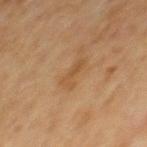Imaged during a routine full-body skin examination; the lesion was not biopsied and no histopathology is available. Located on the mid back. A roughly 15 mm field-of-view crop from a total-body skin photograph. A male patient, roughly 65 years of age. An algorithmic analysis of the crop reported a footprint of about 4.5 mm² and two-axis asymmetry of about 0.4. The software also gave a mean CIELAB color near L≈44 a*≈17 b*≈33, about 6 CIELAB-L* units darker than the surrounding skin, and a lesion-to-skin contrast of about 5.5 (normalized; higher = more distinct). The analysis additionally found a border-irregularity rating of about 5/10, a color-variation rating of about 1.5/10, and radial color variation of about 0.5. The analysis additionally found an automated nevus-likeness rating near 0 out of 100 and a detector confidence of about 100 out of 100 that the crop contains a lesion. Approximately 3.5 mm at its widest. Imaged with cross-polarized lighting.